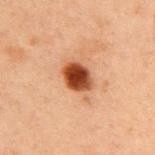Captured under cross-polarized illumination. The lesion is on the upper back. An algorithmic analysis of the crop reported a lesion area of about 7 mm², an outline eccentricity of about 0.65 (0 = round, 1 = elongated), and two-axis asymmetry of about 0.15. The software also gave a mean CIELAB color near L≈35 a*≈24 b*≈31, a lesion–skin lightness drop of about 18, and a normalized border contrast of about 14.5. The analysis additionally found border irregularity of about 1.5 on a 0–10 scale, a color-variation rating of about 4.5/10, and peripheral color asymmetry of about 1. Longest diameter approximately 3.5 mm. A lesion tile, about 15 mm wide, cut from a 3D total-body photograph. A male subject, aged approximately 60.